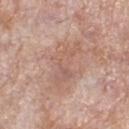biopsy status: no biopsy performed (imaged during a skin exam) | subject: female, in their 70s | site: the right lower leg | tile lighting: white-light | lesion diameter: about 6 mm | acquisition: total-body-photography crop, ~15 mm field of view | image-analysis metrics: a lesion color around L≈59 a*≈20 b*≈27 in CIELAB and a lesion–skin lightness drop of about 7.